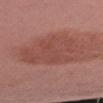Q: Is there a histopathology result?
A: catalogued during a skin exam; not biopsied
Q: Where on the body is the lesion?
A: the right upper arm
Q: Automated lesion metrics?
A: an area of roughly 27 mm², an outline eccentricity of about 0.6 (0 = round, 1 = elongated), and a shape-asymmetry score of about 0.3 (0 = symmetric); border irregularity of about 5 on a 0–10 scale, internal color variation of about 2.5 on a 0–10 scale, and radial color variation of about 1
Q: How large is the lesion?
A: about 6.5 mm
Q: What kind of image is this?
A: ~15 mm tile from a whole-body skin photo
Q: What are the patient's age and sex?
A: male, aged 38 to 42